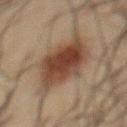The lesion was tiled from a total-body skin photograph and was not biopsied.
A region of skin cropped from a whole-body photographic capture, roughly 15 mm wide.
The lesion is on the chest.
The patient is a male aged approximately 60.